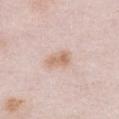| feature | finding |
|---|---|
| workup | imaged on a skin check; not biopsied |
| illumination | white-light |
| site | the chest |
| lesion size | ≈3 mm |
| imaging modality | total-body-photography crop, ~15 mm field of view |
| patient | female, aged 63–67 |